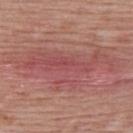Part of a total-body skin-imaging series; this lesion was reviewed on a skin check and was not flagged for biopsy. From the upper back. Captured under white-light illumination. A 15 mm close-up tile from a total-body photography series done for melanoma screening. Approximately 13 mm at its widest. The patient is a female approximately 55 years of age.